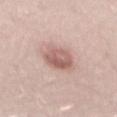Imaged during a routine full-body skin examination; the lesion was not biopsied and no histopathology is available. The lesion is on the mid back. About 4.5 mm across. An algorithmic analysis of the crop reported a shape eccentricity near 0.8 and two-axis asymmetry of about 0.25. The software also gave an average lesion color of about L≈60 a*≈20 b*≈23 (CIELAB), a lesion–skin lightness drop of about 12, and a normalized lesion–skin contrast near 8. And it measured a border-irregularity rating of about 2.5/10, internal color variation of about 5.5 on a 0–10 scale, and peripheral color asymmetry of about 2. A roughly 15 mm field-of-view crop from a total-body skin photograph. A male subject, aged approximately 25. Captured under white-light illumination.